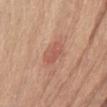No biopsy was performed on this lesion — it was imaged during a full skin examination and was not determined to be concerning. The total-body-photography lesion software estimated a footprint of about 6 mm² and a symmetry-axis asymmetry near 0.15. Measured at roughly 3 mm in maximum diameter. A male patient aged 68 to 72. The lesion is located on the front of the torso. This is a white-light tile. Cropped from a whole-body photographic skin survey; the tile spans about 15 mm.